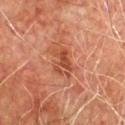The lesion was tiled from a total-body skin photograph and was not biopsied. Captured under cross-polarized illumination. A male patient approximately 75 years of age. An algorithmic analysis of the crop reported a lesion area of about 7.5 mm², a shape eccentricity near 0.8, and a shape-asymmetry score of about 0.25 (0 = symmetric). It also reported a lesion color around L≈41 a*≈25 b*≈30 in CIELAB, about 8 CIELAB-L* units darker than the surrounding skin, and a normalized border contrast of about 6.5. The software also gave a border-irregularity rating of about 3.5/10, internal color variation of about 3.5 on a 0–10 scale, and peripheral color asymmetry of about 1. It also reported lesion-presence confidence of about 100/100. The recorded lesion diameter is about 4 mm. On the chest. A roughly 15 mm field-of-view crop from a total-body skin photograph.